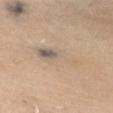Q: How was this image acquired?
A: total-body-photography crop, ~15 mm field of view
Q: Patient demographics?
A: female, roughly 70 years of age
Q: How large is the lesion?
A: about 6 mm
Q: What is the anatomic site?
A: the chest
Q: Automated lesion metrics?
A: an area of roughly 12 mm² and a shape eccentricity near 0.9; a lesion color around L≈63 a*≈11 b*≈27 in CIELAB, roughly 7 lightness units darker than nearby skin, and a lesion-to-skin contrast of about 5 (normalized; higher = more distinct); a border-irregularity index near 4/10, internal color variation of about 10 on a 0–10 scale, and peripheral color asymmetry of about 4; an automated nevus-likeness rating near 0 out of 100 and lesion-presence confidence of about 75/100
Q: What lighting was used for the tile?
A: white-light illumination